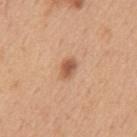<tbp_lesion>
<biopsy_status>not biopsied; imaged during a skin examination</biopsy_status>
<image>
  <source>total-body photography crop</source>
  <field_of_view_mm>15</field_of_view_mm>
</image>
<lighting>white-light</lighting>
<patient>
  <sex>male</sex>
  <age_approx>50</age_approx>
</patient>
<site>mid back</site>
<lesion_size>
  <long_diameter_mm_approx>2.5</long_diameter_mm_approx>
</lesion_size>
<automated_metrics>
  <eccentricity>0.75</eccentricity>
  <cielab_L>57</cielab_L>
  <cielab_a>23</cielab_a>
  <cielab_b>34</cielab_b>
  <vs_skin_contrast_norm>8.0</vs_skin_contrast_norm>
</automated_metrics>
</tbp_lesion>